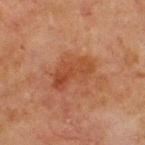Part of a total-body skin-imaging series; this lesion was reviewed on a skin check and was not flagged for biopsy. This is a cross-polarized tile. The lesion-visualizer software estimated an average lesion color of about L≈37 a*≈22 b*≈30 (CIELAB) and a normalized border contrast of about 6.5. It also reported border irregularity of about 4 on a 0–10 scale and radial color variation of about 1.5. The analysis additionally found a nevus-likeness score of about 0/100 and lesion-presence confidence of about 100/100. A 15 mm close-up tile from a total-body photography series done for melanoma screening. A male patient aged 63–67. The lesion is located on the front of the torso. Longest diameter approximately 5.5 mm.